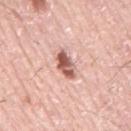This lesion was catalogued during total-body skin photography and was not selected for biopsy.
Approximately 3.5 mm at its widest.
Captured under white-light illumination.
A male subject, aged 73 to 77.
The lesion is located on the back.
The total-body-photography lesion software estimated a lesion area of about 5 mm² and a shape eccentricity near 0.85.
A region of skin cropped from a whole-body photographic capture, roughly 15 mm wide.